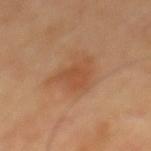<case>
  <biopsy_status>not biopsied; imaged during a skin examination</biopsy_status>
  <patient>
    <sex>male</sex>
    <age_approx>65</age_approx>
  </patient>
  <lighting>cross-polarized</lighting>
  <site>mid back</site>
  <image>
    <source>total-body photography crop</source>
    <field_of_view_mm>15</field_of_view_mm>
  </image>
  <lesion_size>
    <long_diameter_mm_approx>4.5</long_diameter_mm_approx>
  </lesion_size>
</case>